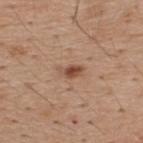{
  "biopsy_status": "not biopsied; imaged during a skin examination",
  "patient": {
    "sex": "male",
    "age_approx": 40
  },
  "site": "upper back",
  "image": {
    "source": "total-body photography crop",
    "field_of_view_mm": 15
  }
}